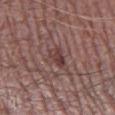The lesion was tiled from a total-body skin photograph and was not biopsied.
Longest diameter approximately 3 mm.
Cropped from a total-body skin-imaging series; the visible field is about 15 mm.
Captured under white-light illumination.
A male subject in their mid- to late 60s.
From the left thigh.
The lesion-visualizer software estimated a lesion area of about 4 mm² and two-axis asymmetry of about 0.25. The software also gave a border-irregularity index near 2.5/10, a within-lesion color-variation index near 4/10, and a peripheral color-asymmetry measure near 1.5. And it measured lesion-presence confidence of about 90/100.